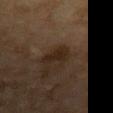notes = no biopsy performed (imaged during a skin exam)
patient = female, roughly 60 years of age
anatomic site = the left forearm
acquisition = total-body-photography crop, ~15 mm field of view